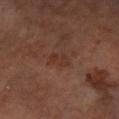notes: catalogued during a skin exam; not biopsied
size: about 2.5 mm
patient: female, roughly 65 years of age
acquisition: total-body-photography crop, ~15 mm field of view
site: the left forearm
lighting: cross-polarized illumination